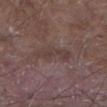notes: imaged on a skin check; not biopsied
lesion size: ~4.5 mm (longest diameter)
patient: male, about 65 years old
image: total-body-photography crop, ~15 mm field of view
site: the arm
automated metrics: an area of roughly 7.5 mm² and a shape eccentricity near 0.85; a lesion color around L≈39 a*≈15 b*≈19 in CIELAB, roughly 6 lightness units darker than nearby skin, and a normalized lesion–skin contrast near 5; border irregularity of about 5 on a 0–10 scale, a color-variation rating of about 2/10, and peripheral color asymmetry of about 0.5
tile lighting: white-light illumination